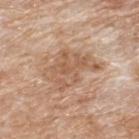No biopsy was performed on this lesion — it was imaged during a full skin examination and was not determined to be concerning.